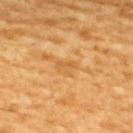Clinical impression: Part of a total-body skin-imaging series; this lesion was reviewed on a skin check and was not flagged for biopsy. Clinical summary: A roughly 15 mm field-of-view crop from a total-body skin photograph. The recorded lesion diameter is about 2.5 mm. Imaged with cross-polarized lighting. The lesion is located on the upper back. The subject is a male aged 58 to 62. Automated image analysis of the tile measured an outline eccentricity of about 0.8 (0 = round, 1 = elongated) and two-axis asymmetry of about 0.45. The analysis additionally found border irregularity of about 4 on a 0–10 scale, internal color variation of about 0 on a 0–10 scale, and peripheral color asymmetry of about 0. The software also gave a classifier nevus-likeness of about 0/100.